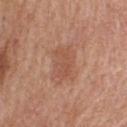workup: catalogued during a skin exam; not biopsied
image: 15 mm crop, total-body photography
diameter: about 4 mm
anatomic site: the upper back
subject: male, about 60 years old
lighting: white-light